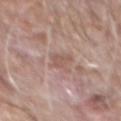biopsy_status: not biopsied; imaged during a skin examination
lesion_size:
  long_diameter_mm_approx: 3.0
lighting: white-light
patient:
  sex: male
  age_approx: 60
image:
  source: total-body photography crop
  field_of_view_mm: 15
automated_metrics:
  eccentricity: 0.9
  shape_asymmetry: 0.5
site: back